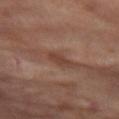This lesion was catalogued during total-body skin photography and was not selected for biopsy.
The lesion is on the left thigh.
A lesion tile, about 15 mm wide, cut from a 3D total-body photograph.
A female patient aged around 55.
Measured at roughly 2.5 mm in maximum diameter.